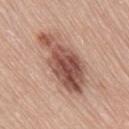Clinical summary:
The subject is a female in their mid-60s. On the right thigh. A 15 mm close-up tile from a total-body photography series done for melanoma screening. An algorithmic analysis of the crop reported a shape eccentricity near 0.9 and a symmetry-axis asymmetry near 0.25. And it measured border irregularity of about 4 on a 0–10 scale and peripheral color asymmetry of about 3.
Conclusion:
Histopathology of the biopsied lesion showed an invasive melanoma arising in association with a nevus, Breslow thickness 0.35 mm and mitotic rate <1/mm².